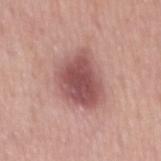Part of a total-body skin-imaging series; this lesion was reviewed on a skin check and was not flagged for biopsy.
A male subject, roughly 65 years of age.
A close-up tile cropped from a whole-body skin photograph, about 15 mm across.
The total-body-photography lesion software estimated a footprint of about 21 mm² and two-axis asymmetry of about 0.2. The analysis additionally found a border-irregularity index near 2.5/10, internal color variation of about 4.5 on a 0–10 scale, and peripheral color asymmetry of about 1.
From the mid back.
The tile uses white-light illumination.